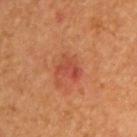No biopsy was performed on this lesion — it was imaged during a full skin examination and was not determined to be concerning. The tile uses cross-polarized illumination. From the left upper arm. A male subject, approximately 55 years of age. A 15 mm close-up extracted from a 3D total-body photography capture. The lesion's longest dimension is about 3 mm.